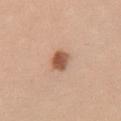Part of a total-body skin-imaging series; this lesion was reviewed on a skin check and was not flagged for biopsy.
A female subject, aged 48–52.
From the front of the torso.
A roughly 15 mm field-of-view crop from a total-body skin photograph.